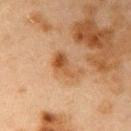Notes:
• workup — total-body-photography surveillance lesion; no biopsy
• image source — 15 mm crop, total-body photography
• lesion diameter — ~4 mm (longest diameter)
• subject — female, aged 38–42
• automated lesion analysis — an area of roughly 6.5 mm², a shape eccentricity near 0.85, and two-axis asymmetry of about 0.5; a border-irregularity rating of about 5.5/10, a within-lesion color-variation index near 5/10, and peripheral color asymmetry of about 1.5
• site — the right upper arm
• tile lighting — cross-polarized